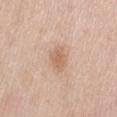Assessment: No biopsy was performed on this lesion — it was imaged during a full skin examination and was not determined to be concerning. Context: The subject is a female in their 60s. Automated tile analysis of the lesion measured a lesion area of about 5 mm², a shape eccentricity near 0.75, and a shape-asymmetry score of about 0.3 (0 = symmetric). This is a white-light tile. Approximately 3 mm at its widest. Located on the abdomen. A region of skin cropped from a whole-body photographic capture, roughly 15 mm wide.